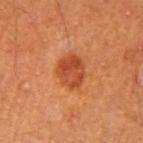notes = catalogued during a skin exam; not biopsied | patient = male, roughly 60 years of age | image source = ~15 mm crop, total-body skin-cancer survey | site = the left upper arm.